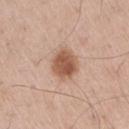Clinical impression: Imaged during a routine full-body skin examination; the lesion was not biopsied and no histopathology is available. Acquisition and patient details: Located on the right thigh. Approximately 3.5 mm at its widest. A 15 mm close-up tile from a total-body photography series done for melanoma screening. A male subject, aged around 60. Captured under white-light illumination. The lesion-visualizer software estimated an average lesion color of about L≈55 a*≈21 b*≈31 (CIELAB), about 14 CIELAB-L* units darker than the surrounding skin, and a normalized lesion–skin contrast near 9.5. The software also gave a nevus-likeness score of about 100/100.